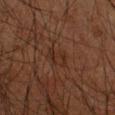<lesion>
<biopsy_status>not biopsied; imaged during a skin examination</biopsy_status>
<lighting>cross-polarized</lighting>
<automated_metrics>
  <vs_skin_darker_L>4.0</vs_skin_darker_L>
  <vs_skin_contrast_norm>5.0</vs_skin_contrast_norm>
  <border_irregularity_0_10>4.0</border_irregularity_0_10>
  <color_variation_0_10>3.0</color_variation_0_10>
  <peripheral_color_asymmetry>1.0</peripheral_color_asymmetry>
  <lesion_detection_confidence_0_100>95</lesion_detection_confidence_0_100>
</automated_metrics>
<patient>
  <sex>male</sex>
  <age_approx>65</age_approx>
</patient>
<lesion_size>
  <long_diameter_mm_approx>3.0</long_diameter_mm_approx>
</lesion_size>
<site>right upper arm</site>
<image>
  <source>total-body photography crop</source>
  <field_of_view_mm>15</field_of_view_mm>
</image>
</lesion>